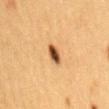Located on the back.
Longest diameter approximately 3 mm.
A female patient about 40 years old.
Cropped from a total-body skin-imaging series; the visible field is about 15 mm.